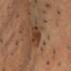{
  "biopsy_status": "not biopsied; imaged during a skin examination",
  "patient": {
    "sex": "male",
    "age_approx": 45
  },
  "image": {
    "source": "total-body photography crop",
    "field_of_view_mm": 15
  },
  "site": "head or neck",
  "lesion_size": {
    "long_diameter_mm_approx": 4.5
  },
  "lighting": "cross-polarized",
  "automated_metrics": {
    "area_mm2_approx": 7.5,
    "eccentricity": 0.85,
    "shape_asymmetry": 0.3,
    "cielab_L": 36,
    "cielab_a": 18,
    "cielab_b": 28,
    "vs_skin_darker_L": 8.0,
    "vs_skin_contrast_norm": 7.5,
    "color_variation_0_10": 3.0,
    "peripheral_color_asymmetry": 1.0
  }
}